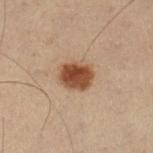Part of a total-body skin-imaging series; this lesion was reviewed on a skin check and was not flagged for biopsy. The subject is a male aged around 55. The total-body-photography lesion software estimated a shape eccentricity near 0.6 and a shape-asymmetry score of about 0.15 (0 = symmetric). The analysis additionally found a lesion color around L≈38 a*≈18 b*≈27 in CIELAB and a lesion-to-skin contrast of about 11.5 (normalized; higher = more distinct). Longest diameter approximately 3.5 mm. The tile uses cross-polarized illumination. This image is a 15 mm lesion crop taken from a total-body photograph. Located on the left lower leg.